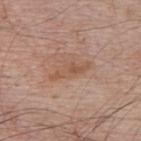Case summary:
* follow-up — total-body-photography surveillance lesion; no biopsy
* location — the upper back
* image source — total-body-photography crop, ~15 mm field of view
* subject — male, roughly 65 years of age
* diameter — about 5 mm